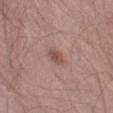biopsy status = catalogued during a skin exam; not biopsied
acquisition = ~15 mm tile from a whole-body skin photo
anatomic site = the left thigh
subject = male, approximately 55 years of age